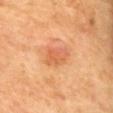Impression: The lesion was photographed on a routine skin check and not biopsied; there is no pathology result.